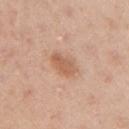The lesion was tiled from a total-body skin photograph and was not biopsied.
The lesion is on the arm.
A female patient, aged approximately 40.
A lesion tile, about 15 mm wide, cut from a 3D total-body photograph.
The lesion's longest dimension is about 3.5 mm.